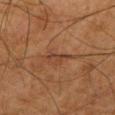The lesion was photographed on a routine skin check and not biopsied; there is no pathology result. A male patient in their 80s. On the right thigh. Automated image analysis of the tile measured a footprint of about 4 mm², an eccentricity of roughly 0.9, and two-axis asymmetry of about 0.55. And it measured an average lesion color of about L≈31 a*≈17 b*≈25 (CIELAB) and a normalized lesion–skin contrast near 6. It also reported a nevus-likeness score of about 0/100. The tile uses cross-polarized illumination. A 15 mm close-up tile from a total-body photography series done for melanoma screening.